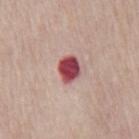Findings:
• notes: total-body-photography surveillance lesion; no biopsy
• site: the chest
• image: 15 mm crop, total-body photography
• illumination: white-light illumination
• patient: male, in their mid-80s or older
• diameter: ≈3 mm
• image-analysis metrics: an area of roughly 6.5 mm², an eccentricity of roughly 0.6, and two-axis asymmetry of about 0.2; a nevus-likeness score of about 0/100